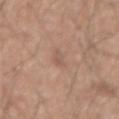<record>
<biopsy_status>not biopsied; imaged during a skin examination</biopsy_status>
<patient>
  <sex>male</sex>
  <age_approx>55</age_approx>
</patient>
<image>
  <source>total-body photography crop</source>
  <field_of_view_mm>15</field_of_view_mm>
</image>
<lighting>white-light</lighting>
</record>